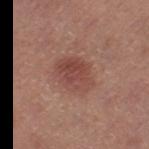Imaged with white-light lighting.
The lesion-visualizer software estimated an area of roughly 11 mm², an eccentricity of roughly 0.6, and two-axis asymmetry of about 0.2. It also reported a border-irregularity index near 2/10 and a color-variation rating of about 4.5/10.
On the left thigh.
A female subject aged 38 to 42.
Approximately 4.5 mm at its widest.
A 15 mm close-up tile from a total-body photography series done for melanoma screening.